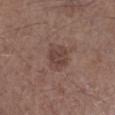<tbp_lesion>
<biopsy_status>not biopsied; imaged during a skin examination</biopsy_status>
<image>
  <source>total-body photography crop</source>
  <field_of_view_mm>15</field_of_view_mm>
</image>
<patient>
  <sex>male</sex>
  <age_approx>60</age_approx>
</patient>
<site>left lower leg</site>
</tbp_lesion>